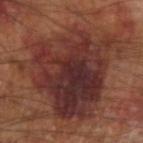Findings:
* biopsy status: imaged on a skin check; not biopsied
* image-analysis metrics: a lesion area of about 70 mm², an outline eccentricity of about 0.45 (0 = round, 1 = elongated), and a symmetry-axis asymmetry near 0.4; an automated nevus-likeness rating near 0 out of 100 and a lesion-detection confidence of about 100/100
* body site: the arm
* image source: ~15 mm crop, total-body skin-cancer survey
* lighting: cross-polarized
* lesion size: ~11 mm (longest diameter)
* patient: male, in their mid- to late 60s